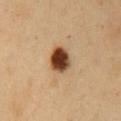No biopsy was performed on this lesion — it was imaged during a full skin examination and was not determined to be concerning.
The lesion is located on the chest.
Automated tile analysis of the lesion measured an outline eccentricity of about 0.75 (0 = round, 1 = elongated) and a symmetry-axis asymmetry near 0.15. It also reported a nevus-likeness score of about 100/100 and a lesion-detection confidence of about 100/100.
This is a cross-polarized tile.
A 15 mm crop from a total-body photograph taken for skin-cancer surveillance.
The lesion's longest dimension is about 4 mm.
A male subject about 50 years old.